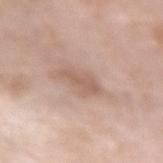Imaged during a routine full-body skin examination; the lesion was not biopsied and no histopathology is available. Cropped from a total-body skin-imaging series; the visible field is about 15 mm. A female subject in their mid-70s. From the left forearm. The total-body-photography lesion software estimated an average lesion color of about L≈59 a*≈18 b*≈27 (CIELAB), a lesion–skin lightness drop of about 8, and a normalized border contrast of about 6. It also reported a border-irregularity rating of about 4/10, internal color variation of about 1 on a 0–10 scale, and radial color variation of about 0.5. The analysis additionally found lesion-presence confidence of about 100/100. The lesion's longest dimension is about 3.5 mm.